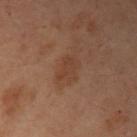<case>
  <biopsy_status>not biopsied; imaged during a skin examination</biopsy_status>
  <image>
    <source>total-body photography crop</source>
    <field_of_view_mm>15</field_of_view_mm>
  </image>
  <lesion_size>
    <long_diameter_mm_approx>3.5</long_diameter_mm_approx>
  </lesion_size>
  <lighting>cross-polarized</lighting>
  <site>left arm</site>
  <patient>
    <sex>female</sex>
    <age_approx>55</age_approx>
  </patient>
</case>